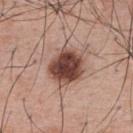Notes:
- biopsy status: imaged on a skin check; not biopsied
- lighting: white-light
- image source: 15 mm crop, total-body photography
- subject: male, roughly 65 years of age
- anatomic site: the back
- size: about 4.5 mm